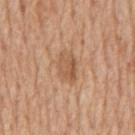{
  "biopsy_status": "not biopsied; imaged during a skin examination",
  "site": "mid back",
  "lighting": "white-light",
  "automated_metrics": {
    "cielab_L": 56,
    "cielab_a": 21,
    "cielab_b": 34,
    "vs_skin_darker_L": 9.0,
    "vs_skin_contrast_norm": 6.5
  },
  "image": {
    "source": "total-body photography crop",
    "field_of_view_mm": 15
  },
  "patient": {
    "sex": "male",
    "age_approx": 65
  }
}